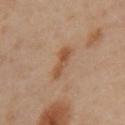follow-up — imaged on a skin check; not biopsied
automated metrics — a lesion color around L≈52 a*≈21 b*≈34 in CIELAB; a classifier nevus-likeness of about 30/100 and a lesion-detection confidence of about 100/100
lesion size — about 4 mm
site — the left upper arm
subject — female, about 40 years old
illumination — cross-polarized
imaging modality — 15 mm crop, total-body photography